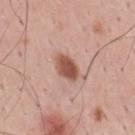No biopsy was performed on this lesion — it was imaged during a full skin examination and was not determined to be concerning. About 3.5 mm across. Located on the mid back. A male patient, aged 33 to 37. A lesion tile, about 15 mm wide, cut from a 3D total-body photograph. Imaged with white-light lighting.